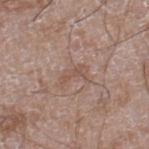notes = no biopsy performed (imaged during a skin exam) | diameter = ~3 mm (longest diameter) | image = ~15 mm crop, total-body skin-cancer survey | subject = male, aged approximately 60 | illumination = white-light illumination | location = the right lower leg.